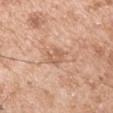The lesion was photographed on a routine skin check and not biopsied; there is no pathology result. The patient is a male aged around 55. Located on the left upper arm. Cropped from a whole-body photographic skin survey; the tile spans about 15 mm.